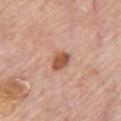Acquisition and patient details: Automated tile analysis of the lesion measured an area of roughly 5 mm² and a shape eccentricity near 0.7. The software also gave roughly 13 lightness units darker than nearby skin and a lesion-to-skin contrast of about 9 (normalized; higher = more distinct). The analysis additionally found border irregularity of about 1.5 on a 0–10 scale, internal color variation of about 2.5 on a 0–10 scale, and peripheral color asymmetry of about 1. The lesion is on the chest. A close-up tile cropped from a whole-body skin photograph, about 15 mm across. Measured at roughly 3 mm in maximum diameter. This is a white-light tile. The patient is a male roughly 80 years of age.